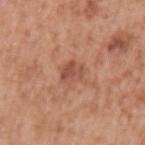  lighting: white-light
  lesion_size:
    long_diameter_mm_approx: 3.0
  patient:
    sex: male
    age_approx: 50
  site: left upper arm
  image:
    source: total-body photography crop
    field_of_view_mm: 15